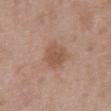Clinical impression:
Recorded during total-body skin imaging; not selected for excision or biopsy.
Clinical summary:
Automated tile analysis of the lesion measured a footprint of about 6.5 mm², an eccentricity of roughly 0.4, and a shape-asymmetry score of about 0.1 (0 = symmetric). And it measured a border-irregularity rating of about 1.5/10 and a color-variation rating of about 1.5/10. And it measured an automated nevus-likeness rating near 0 out of 100 and a lesion-detection confidence of about 100/100. Captured under white-light illumination. Cropped from a whole-body photographic skin survey; the tile spans about 15 mm. The patient is a male aged approximately 50. The lesion's longest dimension is about 3 mm. Located on the abdomen.